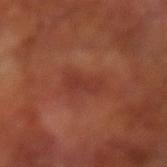This lesion was catalogued during total-body skin photography and was not selected for biopsy.
Approximately 4 mm at its widest.
A male patient aged 63 to 67.
A 15 mm crop from a total-body photograph taken for skin-cancer surveillance.
The lesion is located on the left arm.